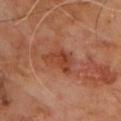follow-up=catalogued during a skin exam; not biopsied | lesion diameter=~3.5 mm (longest diameter) | body site=the upper back | lighting=cross-polarized illumination | subject=male, roughly 60 years of age | imaging modality=~15 mm crop, total-body skin-cancer survey.